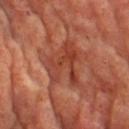Part of a total-body skin-imaging series; this lesion was reviewed on a skin check and was not flagged for biopsy.
The subject is a male roughly 65 years of age.
This image is a 15 mm lesion crop taken from a total-body photograph.
The lesion is on the front of the torso.
Imaged with cross-polarized lighting.